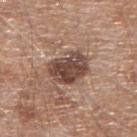notes = no biopsy performed (imaged during a skin exam); image source = ~15 mm tile from a whole-body skin photo; lesion size = about 4.5 mm; body site = the right upper arm; illumination = white-light illumination; subject = male, roughly 65 years of age.